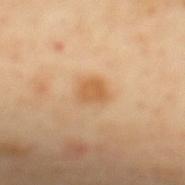Q: Was a biopsy performed?
A: no biopsy performed (imaged during a skin exam)
Q: How was the tile lit?
A: cross-polarized
Q: What is the imaging modality?
A: 15 mm crop, total-body photography
Q: What is the anatomic site?
A: the mid back
Q: How large is the lesion?
A: about 3 mm
Q: What are the patient's age and sex?
A: male, in their mid-60s
Q: What did automated image analysis measure?
A: a normalized border contrast of about 7; border irregularity of about 2 on a 0–10 scale and peripheral color asymmetry of about 1